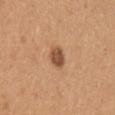Case summary:
– biopsy status — no biopsy performed (imaged during a skin exam)
– image source — total-body-photography crop, ~15 mm field of view
– tile lighting — white-light illumination
– patient — female, approximately 45 years of age
– anatomic site — the left upper arm
– image-analysis metrics — a border-irregularity rating of about 1.5/10 and radial color variation of about 1.5; a classifier nevus-likeness of about 85/100 and a detector confidence of about 100 out of 100 that the crop contains a lesion
– lesion diameter — ≈2.5 mm